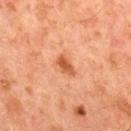Findings:
- biopsy status — no biopsy performed (imaged during a skin exam)
- subject — male, roughly 65 years of age
- lesion diameter — about 2.5 mm
- imaging modality — total-body-photography crop, ~15 mm field of view
- lighting — cross-polarized
- body site — the chest
- automated metrics — an outline eccentricity of about 0.8 (0 = round, 1 = elongated) and a shape-asymmetry score of about 0.2 (0 = symmetric); a border-irregularity rating of about 2/10; a nevus-likeness score of about 95/100 and a lesion-detection confidence of about 100/100